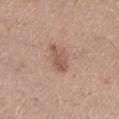{
  "biopsy_status": "not biopsied; imaged during a skin examination",
  "site": "left lower leg",
  "automated_metrics": {
    "cielab_L": 55,
    "cielab_a": 19,
    "cielab_b": 28,
    "vs_skin_darker_L": 9.0,
    "vs_skin_contrast_norm": 6.5
  },
  "image": {
    "source": "total-body photography crop",
    "field_of_view_mm": 15
  },
  "patient": {
    "sex": "female",
    "age_approx": 30
  }
}